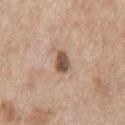Case summary:
• notes: catalogued during a skin exam; not biopsied
• automated metrics: an outline eccentricity of about 0.7 (0 = round, 1 = elongated) and two-axis asymmetry of about 0.25; an average lesion color of about L≈52 a*≈19 b*≈29 (CIELAB); an automated nevus-likeness rating near 95 out of 100 and a detector confidence of about 100 out of 100 that the crop contains a lesion
• body site: the chest
• subject: male, aged 63 to 67
• image: total-body-photography crop, ~15 mm field of view
• tile lighting: white-light